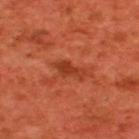biopsy status: no biopsy performed (imaged during a skin exam)
illumination: cross-polarized illumination
automated lesion analysis: a lesion area of about 4.5 mm², an eccentricity of roughly 0.9, and two-axis asymmetry of about 0.4; a mean CIELAB color near L≈38 a*≈33 b*≈37 and about 8 CIELAB-L* units darker than the surrounding skin
subject: male, approximately 60 years of age
diameter: ~3.5 mm (longest diameter)
anatomic site: the upper back
image: ~15 mm tile from a whole-body skin photo